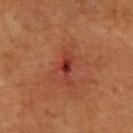A 15 mm close-up tile from a total-body photography series done for melanoma screening. A female subject, roughly 60 years of age. Located on the left forearm. Longest diameter approximately 4 mm.